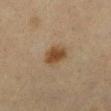follow-up=imaged on a skin check; not biopsied
imaging modality=~15 mm crop, total-body skin-cancer survey
anatomic site=the left lower leg
lighting=cross-polarized
subject=female, aged around 55